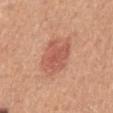• biopsy status · no biopsy performed (imaged during a skin exam)
• automated lesion analysis · an average lesion color of about L≈57 a*≈27 b*≈31 (CIELAB), roughly 9 lightness units darker than nearby skin, and a normalized lesion–skin contrast near 6; a border-irregularity index near 2/10, internal color variation of about 3 on a 0–10 scale, and a peripheral color-asymmetry measure near 1; a nevus-likeness score of about 85/100
• size · ≈5 mm
• imaging modality · ~15 mm tile from a whole-body skin photo
• location · the mid back
• tile lighting · white-light
• subject · female, aged 53–57